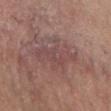Assessment: The lesion was tiled from a total-body skin photograph and was not biopsied. Acquisition and patient details: Longest diameter approximately 6 mm. A roughly 15 mm field-of-view crop from a total-body skin photograph. The lesion-visualizer software estimated an area of roughly 15 mm², an eccentricity of roughly 0.8, and a shape-asymmetry score of about 0.35 (0 = symmetric). It also reported a lesion-detection confidence of about 90/100. A female subject in their mid-40s. Located on the chest. Captured under white-light illumination.